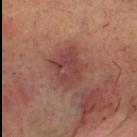Assessment: Recorded during total-body skin imaging; not selected for excision or biopsy. Acquisition and patient details: The lesion is on the head or neck. A male patient, aged approximately 55. A roughly 15 mm field-of-view crop from a total-body skin photograph.